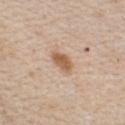The lesion was tiled from a total-body skin photograph and was not biopsied. A female subject, aged around 45. The lesion's longest dimension is about 3.5 mm. The lesion-visualizer software estimated a footprint of about 5.5 mm², an eccentricity of roughly 0.8, and a symmetry-axis asymmetry near 0.2. The software also gave roughly 12 lightness units darker than nearby skin. Cropped from a whole-body photographic skin survey; the tile spans about 15 mm. The lesion is located on the front of the torso.